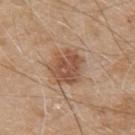Assessment:
Imaged during a routine full-body skin examination; the lesion was not biopsied and no histopathology is available.
Clinical summary:
Measured at roughly 4.5 mm in maximum diameter. A region of skin cropped from a whole-body photographic capture, roughly 15 mm wide. Imaged with white-light lighting. A male patient, roughly 80 years of age. The lesion is on the upper back.